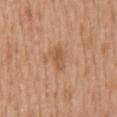The lesion was tiled from a total-body skin photograph and was not biopsied.
Imaged with white-light lighting.
The patient is a female aged 38–42.
The lesion is on the mid back.
The recorded lesion diameter is about 3 mm.
A 15 mm close-up tile from a total-body photography series done for melanoma screening.